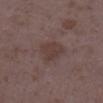This lesion was catalogued during total-body skin photography and was not selected for biopsy. The tile uses white-light illumination. From the right lower leg. A region of skin cropped from a whole-body photographic capture, roughly 15 mm wide. The lesion-visualizer software estimated a lesion area of about 9 mm² and an outline eccentricity of about 0.4 (0 = round, 1 = elongated). The analysis additionally found a border-irregularity index near 2.5/10, a within-lesion color-variation index near 2/10, and a peripheral color-asymmetry measure near 0.5. A female patient, in their mid- to late 30s. The lesion's longest dimension is about 3.5 mm.